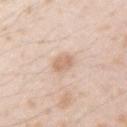Part of a total-body skin-imaging series; this lesion was reviewed on a skin check and was not flagged for biopsy.
A lesion tile, about 15 mm wide, cut from a 3D total-body photograph.
Measured at roughly 2.5 mm in maximum diameter.
This is a white-light tile.
The patient is a male aged approximately 25.
The lesion is on the right upper arm.